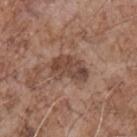Q: How was this image acquired?
A: total-body-photography crop, ~15 mm field of view
Q: What lighting was used for the tile?
A: white-light illumination
Q: Where on the body is the lesion?
A: the chest
Q: What are the patient's age and sex?
A: male, aged 68–72